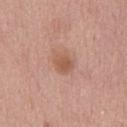biopsy status: imaged on a skin check; not biopsied
diameter: ≈2.5 mm
illumination: white-light illumination
automated lesion analysis: an average lesion color of about L≈55 a*≈22 b*≈30 (CIELAB), roughly 9 lightness units darker than nearby skin, and a normalized lesion–skin contrast near 7; a border-irregularity rating of about 1.5/10, a within-lesion color-variation index near 2/10, and peripheral color asymmetry of about 0.5
patient: male, aged approximately 75
image: ~15 mm tile from a whole-body skin photo
body site: the chest